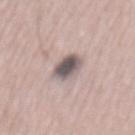Notes:
- notes — total-body-photography surveillance lesion; no biopsy
- subject — male, approximately 55 years of age
- acquisition — 15 mm crop, total-body photography